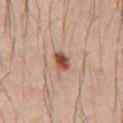This lesion was catalogued during total-body skin photography and was not selected for biopsy.
This is a white-light tile.
The recorded lesion diameter is about 2.5 mm.
The total-body-photography lesion software estimated a lesion color around L≈51 a*≈22 b*≈29 in CIELAB, a lesion–skin lightness drop of about 15, and a normalized border contrast of about 10.5. It also reported a classifier nevus-likeness of about 100/100.
A male patient, roughly 45 years of age.
A 15 mm crop from a total-body photograph taken for skin-cancer surveillance.
Located on the chest.